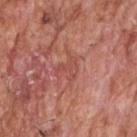notes=catalogued during a skin exam; not biopsied | imaging modality=total-body-photography crop, ~15 mm field of view | subject=male, roughly 60 years of age | body site=the head or neck.